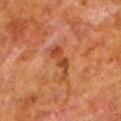biopsy status = catalogued during a skin exam; not biopsied
automated lesion analysis = a within-lesion color-variation index near 2.5/10 and radial color variation of about 0.5
subject = male, aged around 80
site = the left lower leg
tile lighting = cross-polarized illumination
size = about 4.5 mm
image = ~15 mm tile from a whole-body skin photo